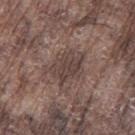workup = imaged on a skin check; not biopsied | lesion diameter = about 4.5 mm | automated lesion analysis = a footprint of about 10 mm², a shape eccentricity near 0.75, and two-axis asymmetry of about 0.3; a mean CIELAB color near L≈41 a*≈14 b*≈19, about 8 CIELAB-L* units darker than the surrounding skin, and a normalized border contrast of about 7; internal color variation of about 3 on a 0–10 scale; a classifier nevus-likeness of about 0/100 and a detector confidence of about 80 out of 100 that the crop contains a lesion | image source = total-body-photography crop, ~15 mm field of view | lighting = white-light | site = the left thigh | subject = male, roughly 75 years of age.